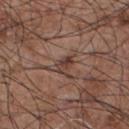Clinical impression:
The lesion was tiled from a total-body skin photograph and was not biopsied.
Context:
Located on the front of the torso. A male subject roughly 55 years of age. Imaged with white-light lighting. Cropped from a whole-body photographic skin survey; the tile spans about 15 mm. The lesion's longest dimension is about 2.5 mm.